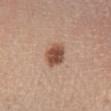Clinical impression: Captured during whole-body skin photography for melanoma surveillance; the lesion was not biopsied. Image and clinical context: From the left lower leg. This is a white-light tile. Cropped from a whole-body photographic skin survey; the tile spans about 15 mm. The total-body-photography lesion software estimated an area of roughly 7 mm², a shape eccentricity near 0.3, and two-axis asymmetry of about 0.15. It also reported a mean CIELAB color near L≈51 a*≈21 b*≈29, a lesion–skin lightness drop of about 14, and a normalized border contrast of about 10. The software also gave a detector confidence of about 100 out of 100 that the crop contains a lesion. About 3 mm across. A male patient in their mid- to late 50s.